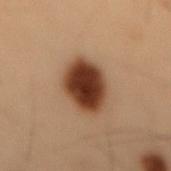Captured during whole-body skin photography for melanoma surveillance; the lesion was not biopsied. An algorithmic analysis of the crop reported an area of roughly 14 mm², a shape eccentricity near 0.8, and two-axis asymmetry of about 0.25. The analysis additionally found an average lesion color of about L≈29 a*≈19 b*≈26 (CIELAB), a lesion–skin lightness drop of about 18, and a normalized border contrast of about 16. The tile uses cross-polarized illumination. A male subject about 55 years old. The lesion is on the lower back. A roughly 15 mm field-of-view crop from a total-body skin photograph. Measured at roughly 5.5 mm in maximum diameter.